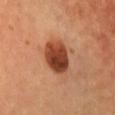No biopsy was performed on this lesion — it was imaged during a full skin examination and was not determined to be concerning.